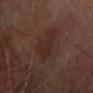The lesion was tiled from a total-body skin photograph and was not biopsied. The lesion is located on the right forearm. A male subject, aged approximately 75. A 15 mm close-up tile from a total-body photography series done for melanoma screening. This is a cross-polarized tile.